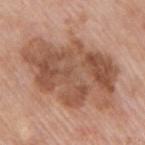Impression: No biopsy was performed on this lesion — it was imaged during a full skin examination and was not determined to be concerning. Image and clinical context: Measured at roughly 12 mm in maximum diameter. The lesion-visualizer software estimated an average lesion color of about L≈53 a*≈21 b*≈30 (CIELAB), a lesion–skin lightness drop of about 12, and a lesion-to-skin contrast of about 8 (normalized; higher = more distinct). A male patient in their mid- to late 50s. This image is a 15 mm lesion crop taken from a total-body photograph. Captured under white-light illumination. The lesion is located on the right upper arm.